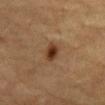Clinical impression:
Recorded during total-body skin imaging; not selected for excision or biopsy.
Acquisition and patient details:
The lesion-visualizer software estimated an average lesion color of about L≈31 a*≈18 b*≈29 (CIELAB) and a normalized border contrast of about 11. It also reported an automated nevus-likeness rating near 100 out of 100 and a detector confidence of about 100 out of 100 that the crop contains a lesion. The patient is a male aged approximately 85 or older. Imaged with cross-polarized lighting. Located on the abdomen. A 15 mm close-up tile from a total-body photography series done for melanoma screening. Measured at roughly 2.5 mm in maximum diameter.